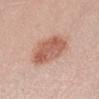{"biopsy_status": "not biopsied; imaged during a skin examination", "patient": {"sex": "female", "age_approx": 30}, "automated_metrics": {"eccentricity": 0.6, "shape_asymmetry": 0.15, "nevus_likeness_0_100": 95}, "image": {"source": "total-body photography crop", "field_of_view_mm": 15}, "site": "abdomen", "lighting": "white-light"}